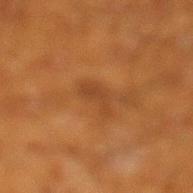{"biopsy_status": "not biopsied; imaged during a skin examination", "site": "leg", "image": {"source": "total-body photography crop", "field_of_view_mm": 15}, "patient": {"sex": "male", "age_approx": 60}}